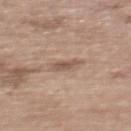workup = catalogued during a skin exam; not biopsied
body site = the mid back
lighting = white-light
acquisition = total-body-photography crop, ~15 mm field of view
automated metrics = an area of roughly 3 mm², an eccentricity of roughly 0.85, and a symmetry-axis asymmetry near 0.3; a mean CIELAB color near L≈54 a*≈17 b*≈26, about 10 CIELAB-L* units darker than the surrounding skin, and a normalized border contrast of about 6.5; border irregularity of about 3 on a 0–10 scale, a color-variation rating of about 1.5/10, and a peripheral color-asymmetry measure near 0.5; a classifier nevus-likeness of about 5/100 and a detector confidence of about 100 out of 100 that the crop contains a lesion
patient = female, aged approximately 65
size = ≈2.5 mm